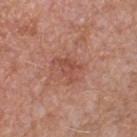From the left thigh. About 3.5 mm across. The tile uses white-light illumination. A lesion tile, about 15 mm wide, cut from a 3D total-body photograph. The lesion-visualizer software estimated a border-irregularity index near 5/10, a within-lesion color-variation index near 3/10, and peripheral color asymmetry of about 1. And it measured an automated nevus-likeness rating near 5 out of 100. A female patient aged approximately 40.